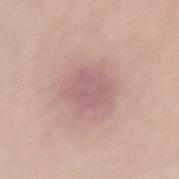| key | value |
|---|---|
| workup | total-body-photography surveillance lesion; no biopsy |
| TBP lesion metrics | an area of roughly 9.5 mm², an eccentricity of roughly 0.65, and two-axis asymmetry of about 0.2; a lesion color around L≈60 a*≈22 b*≈19 in CIELAB, roughly 7 lightness units darker than nearby skin, and a normalized border contrast of about 5.5; lesion-presence confidence of about 100/100 |
| location | the lower back |
| diameter | ~4.5 mm (longest diameter) |
| patient | female, aged around 40 |
| acquisition | ~15 mm tile from a whole-body skin photo |